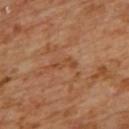<record>
  <biopsy_status>not biopsied; imaged during a skin examination</biopsy_status>
</record>